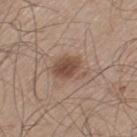Recorded during total-body skin imaging; not selected for excision or biopsy.
A male subject, in their 60s.
A close-up tile cropped from a whole-body skin photograph, about 15 mm across.
From the left thigh.
Approximately 4 mm at its widest.
Captured under white-light illumination.